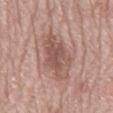Part of a total-body skin-imaging series; this lesion was reviewed on a skin check and was not flagged for biopsy.
A roughly 15 mm field-of-view crop from a total-body skin photograph.
The subject is a male aged approximately 80.
From the mid back.
The tile uses white-light illumination.
Longest diameter approximately 7.5 mm.